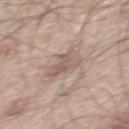Assessment: The lesion was photographed on a routine skin check and not biopsied; there is no pathology result. Context: The lesion-visualizer software estimated a lesion area of about 7.5 mm², a shape eccentricity near 0.6, and two-axis asymmetry of about 0.4. The analysis additionally found an average lesion color of about L≈57 a*≈15 b*≈23 (CIELAB), about 9 CIELAB-L* units darker than the surrounding skin, and a normalized lesion–skin contrast near 6. The software also gave a nevus-likeness score of about 0/100. The lesion is on the mid back. A region of skin cropped from a whole-body photographic capture, roughly 15 mm wide. A male subject, aged around 55. Measured at roughly 3.5 mm in maximum diameter. This is a white-light tile.